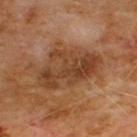  biopsy_status: not biopsied; imaged during a skin examination